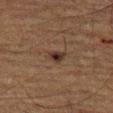Captured during whole-body skin photography for melanoma surveillance; the lesion was not biopsied. The recorded lesion diameter is about 2 mm. This is a cross-polarized tile. From the left thigh. The subject is a male aged approximately 75. A region of skin cropped from a whole-body photographic capture, roughly 15 mm wide.